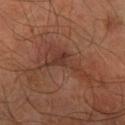The lesion was tiled from a total-body skin photograph and was not biopsied.
Located on the right forearm.
Measured at roughly 6.5 mm in maximum diameter.
A male subject, aged 63–67.
A 15 mm crop from a total-body photograph taken for skin-cancer surveillance.